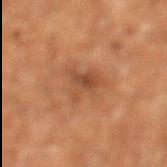workup: total-body-photography surveillance lesion; no biopsy
lesion diameter: about 5 mm
location: the left lower leg
subject: male, aged 58 to 62
lighting: cross-polarized
imaging modality: ~15 mm tile from a whole-body skin photo